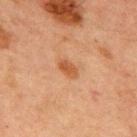Clinical impression: This lesion was catalogued during total-body skin photography and was not selected for biopsy. Context: The lesion-visualizer software estimated a shape eccentricity near 0.8. It also reported border irregularity of about 2.5 on a 0–10 scale, a color-variation rating of about 2.5/10, and a peripheral color-asymmetry measure near 1. A lesion tile, about 15 mm wide, cut from a 3D total-body photograph. Approximately 2.5 mm at its widest. On the chest. Imaged with cross-polarized lighting. A male subject, aged around 65.